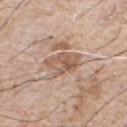The lesion was photographed on a routine skin check and not biopsied; there is no pathology result. This is a white-light tile. This image is a 15 mm lesion crop taken from a total-body photograph. A male patient about 75 years old. The lesion is on the chest.